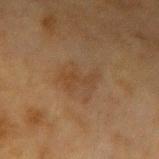Q: Was a biopsy performed?
A: imaged on a skin check; not biopsied
Q: What lighting was used for the tile?
A: cross-polarized
Q: Patient demographics?
A: female, aged 53 to 57
Q: Automated lesion metrics?
A: an area of roughly 11 mm², an outline eccentricity of about 0.6 (0 = round, 1 = elongated), and a shape-asymmetry score of about 0.3 (0 = symmetric); a lesion color around L≈35 a*≈14 b*≈27 in CIELAB, a lesion–skin lightness drop of about 4, and a normalized border contrast of about 4.5; an automated nevus-likeness rating near 0 out of 100 and a lesion-detection confidence of about 100/100
Q: Lesion location?
A: the left forearm
Q: How large is the lesion?
A: about 4 mm
Q: What kind of image is this?
A: ~15 mm crop, total-body skin-cancer survey